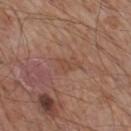  site: right thigh
  patient:
    sex: male
    age_approx: 55
  image:
    source: total-body photography crop
    field_of_view_mm: 15
  lighting: white-light
  automated_metrics:
    cielab_L: 46
    cielab_a: 20
    cielab_b: 28
    border_irregularity_0_10: 3.0
    color_variation_0_10: 0.0
    peripheral_color_asymmetry: 0.0
  lesion_size:
    long_diameter_mm_approx: 1.5